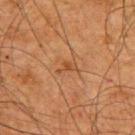Case summary:
* biopsy status — no biopsy performed (imaged during a skin exam)
* acquisition — ~15 mm crop, total-body skin-cancer survey
* site — the upper back
* subject — male, in their mid-60s